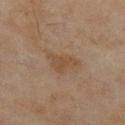<tbp_lesion>
  <site>right lower leg</site>
  <image>
    <source>total-body photography crop</source>
    <field_of_view_mm>15</field_of_view_mm>
  </image>
  <lesion_size>
    <long_diameter_mm_approx>4.0</long_diameter_mm_approx>
  </lesion_size>
  <automated_metrics>
    <vs_skin_darker_L>6.0</vs_skin_darker_L>
    <vs_skin_contrast_norm>6.0</vs_skin_contrast_norm>
    <border_irregularity_0_10>5.0</border_irregularity_0_10>
    <peripheral_color_asymmetry>0.5</peripheral_color_asymmetry>
  </automated_metrics>
  <lighting>cross-polarized</lighting>
  <patient>
    <sex>female</sex>
    <age_approx>60</age_approx>
  </patient>
</tbp_lesion>